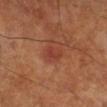Imaged during a routine full-body skin examination; the lesion was not biopsied and no histopathology is available.
The tile uses cross-polarized illumination.
The lesion is located on the right lower leg.
The lesion's longest dimension is about 2.5 mm.
A close-up tile cropped from a whole-body skin photograph, about 15 mm across.
A male subject approximately 70 years of age.
An algorithmic analysis of the crop reported a footprint of about 3 mm² and an eccentricity of roughly 0.8.